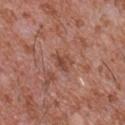follow-up=no biopsy performed (imaged during a skin exam); image=total-body-photography crop, ~15 mm field of view; location=the chest; subject=male, aged around 45; lesion diameter=about 2.5 mm; TBP lesion metrics=an average lesion color of about L≈46 a*≈24 b*≈29 (CIELAB), roughly 8 lightness units darker than nearby skin, and a normalized lesion–skin contrast near 6.5; illumination=white-light.